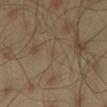| field | value |
|---|---|
| workup | total-body-photography surveillance lesion; no biopsy |
| patient | male, about 45 years old |
| acquisition | ~15 mm crop, total-body skin-cancer survey |
| automated metrics | a lesion area of about 0.5 mm²; border irregularity of about 3.5 on a 0–10 scale, a color-variation rating of about 0/10, and radial color variation of about 0; an automated nevus-likeness rating near 0 out of 100 and lesion-presence confidence of about 65/100 |
| site | the left thigh |
| diameter | about 1 mm |
| lighting | cross-polarized illumination |